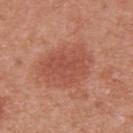Located on the upper back.
Imaged with white-light lighting.
Measured at roughly 6.5 mm in maximum diameter.
The subject is a female roughly 40 years of age.
Cropped from a total-body skin-imaging series; the visible field is about 15 mm.
Histopathological examination showed a compound melanocytic nevus.